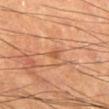notes: catalogued during a skin exam; not biopsied | body site: the left lower leg | TBP lesion metrics: a symmetry-axis asymmetry near 0.3; about 8 CIELAB-L* units darker than the surrounding skin | image: 15 mm crop, total-body photography | subject: male, aged 63 to 67 | diameter: about 1.5 mm.